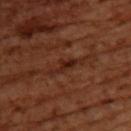subject: female, aged approximately 55; image: ~15 mm tile from a whole-body skin photo; illumination: cross-polarized illumination; anatomic site: the back.